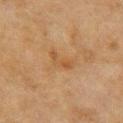The lesion was photographed on a routine skin check and not biopsied; there is no pathology result. The lesion is located on the right upper arm. A female subject aged 58 to 62. This is a cross-polarized tile. A roughly 15 mm field-of-view crop from a total-body skin photograph.